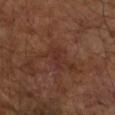{"biopsy_status": "not biopsied; imaged during a skin examination", "site": "right upper arm", "lesion_size": {"long_diameter_mm_approx": 3.0}, "patient": {"sex": "male", "age_approx": 65}, "lighting": "cross-polarized", "image": {"source": "total-body photography crop", "field_of_view_mm": 15}}